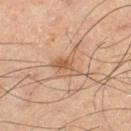Impression: Imaged during a routine full-body skin examination; the lesion was not biopsied and no histopathology is available. Clinical summary: The lesion-visualizer software estimated a lesion area of about 5.5 mm². It also reported roughly 7 lightness units darker than nearby skin and a normalized border contrast of about 6. And it measured a border-irregularity rating of about 6.5/10, internal color variation of about 1.5 on a 0–10 scale, and a peripheral color-asymmetry measure near 0.5. The software also gave a nevus-likeness score of about 30/100 and a lesion-detection confidence of about 100/100. On the right thigh. Cropped from a whole-body photographic skin survey; the tile spans about 15 mm. The patient is a male in their mid-60s. The lesion's longest dimension is about 3.5 mm.